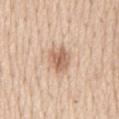Recorded during total-body skin imaging; not selected for excision or biopsy. A male subject about 60 years old. The lesion is on the chest. A 15 mm close-up tile from a total-body photography series done for melanoma screening. Approximately 3.5 mm at its widest. Imaged with white-light lighting.